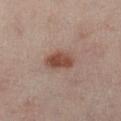follow-up=imaged on a skin check; not biopsied
tile lighting=cross-polarized
automated lesion analysis=a color-variation rating of about 3.5/10 and radial color variation of about 1; an automated nevus-likeness rating near 100 out of 100 and lesion-presence confidence of about 100/100
subject=female, in their mid-50s
imaging modality=15 mm crop, total-body photography
body site=the left lower leg
size=≈3.5 mm